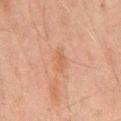The lesion was photographed on a routine skin check and not biopsied; there is no pathology result. The lesion-visualizer software estimated a lesion color around L≈49 a*≈19 b*≈29 in CIELAB, a lesion–skin lightness drop of about 5, and a normalized lesion–skin contrast near 5. It also reported a border-irregularity rating of about 2.5/10, a within-lesion color-variation index near 1.5/10, and a peripheral color-asymmetry measure near 0.5. The software also gave a nevus-likeness score of about 0/100 and a detector confidence of about 100 out of 100 that the crop contains a lesion. About 3 mm across. The patient is a male approximately 45 years of age. This image is a 15 mm lesion crop taken from a total-body photograph. The tile uses cross-polarized illumination. On the mid back.